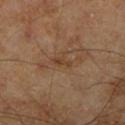Recorded during total-body skin imaging; not selected for excision or biopsy. Measured at roughly 2.5 mm in maximum diameter. A male subject aged 68–72. From the right lower leg. A close-up tile cropped from a whole-body skin photograph, about 15 mm across. The total-body-photography lesion software estimated a lesion color around L≈39 a*≈18 b*≈30 in CIELAB and a lesion–skin lightness drop of about 6. The software also gave a border-irregularity index near 4.5/10, internal color variation of about 0 on a 0–10 scale, and a peripheral color-asymmetry measure near 0. The analysis additionally found a classifier nevus-likeness of about 0/100 and lesion-presence confidence of about 95/100. This is a cross-polarized tile.